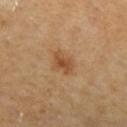Captured during whole-body skin photography for melanoma surveillance; the lesion was not biopsied.